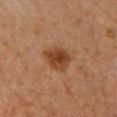notes = no biopsy performed (imaged during a skin exam) | size = ~4 mm (longest diameter) | automated metrics = a nevus-likeness score of about 95/100 and lesion-presence confidence of about 100/100 | subject = female, aged around 40 | location = the chest | image = ~15 mm crop, total-body skin-cancer survey | tile lighting = cross-polarized illumination.